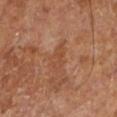Clinical summary:
A 15 mm close-up tile from a total-body photography series done for melanoma screening. The lesion is located on the leg. About 3.5 mm across. A patient in their mid- to late 60s. Captured under cross-polarized illumination.